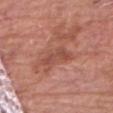Clinical impression:
Part of a total-body skin-imaging series; this lesion was reviewed on a skin check and was not flagged for biopsy.
Acquisition and patient details:
Located on the chest. A region of skin cropped from a whole-body photographic capture, roughly 15 mm wide. A male subject approximately 80 years of age.